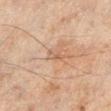The lesion was photographed on a routine skin check and not biopsied; there is no pathology result. A male subject approximately 45 years of age. The lesion's longest dimension is about 2.5 mm. The lesion is on the left lower leg. Cropped from a total-body skin-imaging series; the visible field is about 15 mm.